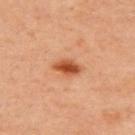Q: Was this lesion biopsied?
A: catalogued during a skin exam; not biopsied
Q: Who is the patient?
A: male, aged 43–47
Q: What kind of image is this?
A: total-body-photography crop, ~15 mm field of view
Q: Lesion location?
A: the upper back